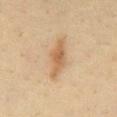workup=catalogued during a skin exam; not biopsied
size=~5 mm (longest diameter)
TBP lesion metrics=a lesion color around L≈56 a*≈17 b*≈34 in CIELAB, roughly 10 lightness units darker than nearby skin, and a normalized border contrast of about 7; border irregularity of about 3.5 on a 0–10 scale, a within-lesion color-variation index near 2.5/10, and peripheral color asymmetry of about 0.5; an automated nevus-likeness rating near 80 out of 100
body site=the abdomen
image source=~15 mm crop, total-body skin-cancer survey
subject=male, approximately 35 years of age